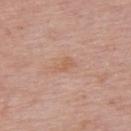biopsy status = imaged on a skin check; not biopsied | subject = female, aged 63–67 | image source = ~15 mm crop, total-body skin-cancer survey | location = the upper back.